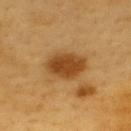Impression: No biopsy was performed on this lesion — it was imaged during a full skin examination and was not determined to be concerning. Background: This is a cross-polarized tile. Approximately 5 mm at its widest. The lesion is on the upper back. Cropped from a total-body skin-imaging series; the visible field is about 15 mm. The total-body-photography lesion software estimated an automated nevus-likeness rating near 100 out of 100 and a detector confidence of about 100 out of 100 that the crop contains a lesion. The patient is a male aged 58–62.